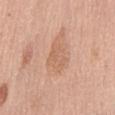This lesion was catalogued during total-body skin photography and was not selected for biopsy. A 15 mm close-up extracted from a 3D total-body photography capture. The lesion-visualizer software estimated an area of roughly 6 mm² and two-axis asymmetry of about 0.35. And it measured a lesion–skin lightness drop of about 7 and a lesion-to-skin contrast of about 4.5 (normalized; higher = more distinct). The analysis additionally found an automated nevus-likeness rating near 0 out of 100 and a detector confidence of about 100 out of 100 that the crop contains a lesion. The lesion is located on the mid back. Captured under white-light illumination. A female subject, aged 63–67.